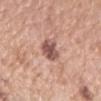Clinical impression: This lesion was catalogued during total-body skin photography and was not selected for biopsy. Background: The tile uses white-light illumination. A region of skin cropped from a whole-body photographic capture, roughly 15 mm wide. The lesion is located on the right forearm. A female patient aged 58–62. The lesion's longest dimension is about 3 mm.